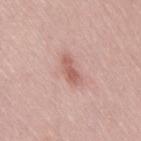| key | value |
|---|---|
| biopsy status | catalogued during a skin exam; not biopsied |
| body site | the right thigh |
| image | ~15 mm crop, total-body skin-cancer survey |
| lighting | white-light |
| patient | female, aged 28 to 32 |